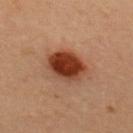A female subject, roughly 35 years of age. This is a cross-polarized tile. A close-up tile cropped from a whole-body skin photograph, about 15 mm across. The lesion is located on the upper back.